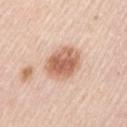Q: Was this lesion biopsied?
A: catalogued during a skin exam; not biopsied
Q: Lesion location?
A: the left upper arm
Q: How was this image acquired?
A: ~15 mm tile from a whole-body skin photo
Q: Patient demographics?
A: male, aged approximately 45
Q: What did automated image analysis measure?
A: an area of roughly 13 mm², an outline eccentricity of about 0.65 (0 = round, 1 = elongated), and a symmetry-axis asymmetry near 0.1; an average lesion color of about L≈63 a*≈22 b*≈31 (CIELAB), a lesion–skin lightness drop of about 14, and a lesion-to-skin contrast of about 9 (normalized; higher = more distinct); a border-irregularity index near 1.5/10 and a peripheral color-asymmetry measure near 1.5; an automated nevus-likeness rating near 100 out of 100 and a lesion-detection confidence of about 100/100